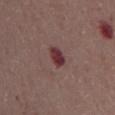Clinical impression:
Recorded during total-body skin imaging; not selected for excision or biopsy.
Acquisition and patient details:
Longest diameter approximately 2.5 mm. From the front of the torso. The subject is a male about 70 years old. A roughly 15 mm field-of-view crop from a total-body skin photograph. Imaged with white-light lighting.